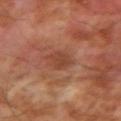biopsy status: catalogued during a skin exam; not biopsied
subject: male, approximately 70 years of age
acquisition: ~15 mm crop, total-body skin-cancer survey
automated metrics: an average lesion color of about L≈42 a*≈24 b*≈30 (CIELAB), a lesion–skin lightness drop of about 7, and a lesion-to-skin contrast of about 6 (normalized; higher = more distinct); a color-variation rating of about 1.5/10
location: the right upper arm
lesion size: ~2.5 mm (longest diameter)
illumination: cross-polarized illumination